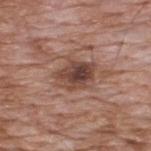<record>
  <biopsy_status>not biopsied; imaged during a skin examination</biopsy_status>
  <patient>
    <sex>male</sex>
    <age_approx>60</age_approx>
  </patient>
  <site>upper back</site>
  <automated_metrics>
    <area_mm2_approx>10.0</area_mm2_approx>
    <eccentricity>0.75</eccentricity>
    <shape_asymmetry>0.25</shape_asymmetry>
    <cielab_L>44</cielab_L>
    <cielab_a>20</cielab_a>
    <cielab_b>25</cielab_b>
    <vs_skin_darker_L>13.0</vs_skin_darker_L>
    <vs_skin_contrast_norm>9.5</vs_skin_contrast_norm>
    <border_irregularity_0_10>2.5</border_irregularity_0_10>
    <peripheral_color_asymmetry>2.5</peripheral_color_asymmetry>
  </automated_metrics>
  <image>
    <source>total-body photography crop</source>
    <field_of_view_mm>15</field_of_view_mm>
  </image>
  <lesion_size>
    <long_diameter_mm_approx>4.0</long_diameter_mm_approx>
  </lesion_size>
</record>